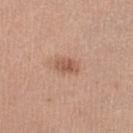workup: no biopsy performed (imaged during a skin exam)
patient: female, approximately 35 years of age
body site: the leg
TBP lesion metrics: a lesion area of about 4.5 mm², an outline eccentricity of about 0.8 (0 = round, 1 = elongated), and a shape-asymmetry score of about 0.3 (0 = symmetric); an average lesion color of about L≈56 a*≈22 b*≈30 (CIELAB), roughly 10 lightness units darker than nearby skin, and a lesion-to-skin contrast of about 7 (normalized; higher = more distinct)
image: total-body-photography crop, ~15 mm field of view
lighting: white-light illumination
lesion diameter: ≈2.5 mm